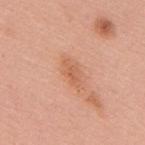biopsy status: no biopsy performed (imaged during a skin exam)
automated metrics: a mean CIELAB color near L≈61 a*≈26 b*≈36, about 8 CIELAB-L* units darker than the surrounding skin, and a lesion-to-skin contrast of about 6 (normalized; higher = more distinct)
patient: female, in their 60s
size: about 3.5 mm
anatomic site: the mid back
image source: ~15 mm crop, total-body skin-cancer survey